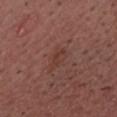Recorded during total-body skin imaging; not selected for excision or biopsy. A close-up tile cropped from a whole-body skin photograph, about 15 mm across. A male subject, aged around 55. The lesion-visualizer software estimated a footprint of about 3.5 mm² and a shape-asymmetry score of about 0.2 (0 = symmetric). It also reported border irregularity of about 2 on a 0–10 scale, a within-lesion color-variation index near 1.5/10, and a peripheral color-asymmetry measure near 0.5. The software also gave a classifier nevus-likeness of about 0/100 and lesion-presence confidence of about 100/100. From the chest. The recorded lesion diameter is about 2.5 mm. Imaged with white-light lighting.